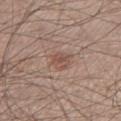The lesion was photographed on a routine skin check and not biopsied; there is no pathology result.
A 15 mm close-up extracted from a 3D total-body photography capture.
The lesion is located on the left lower leg.
A male subject, aged around 60.
Longest diameter approximately 3.5 mm.
The tile uses white-light illumination.
Automated image analysis of the tile measured a color-variation rating of about 2.5/10 and radial color variation of about 1.